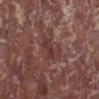Imaged during a routine full-body skin examination; the lesion was not biopsied and no histopathology is available. A roughly 15 mm field-of-view crop from a total-body skin photograph. Located on the leg. An algorithmic analysis of the crop reported about 7 CIELAB-L* units darker than the surrounding skin and a normalized lesion–skin contrast near 6.5. And it measured a border-irregularity index near 3/10 and peripheral color asymmetry of about 0.5. The software also gave a nevus-likeness score of about 0/100 and a detector confidence of about 85 out of 100 that the crop contains a lesion. The patient is a male approximately 65 years of age. Longest diameter approximately 3 mm. The tile uses white-light illumination.